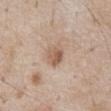Case summary:
- workup: imaged on a skin check; not biopsied
- anatomic site: the abdomen
- subject: male, about 60 years old
- image source: ~15 mm tile from a whole-body skin photo